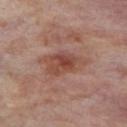{
  "biopsy_status": "not biopsied; imaged during a skin examination",
  "lesion_size": {
    "long_diameter_mm_approx": 4.5
  },
  "site": "leg",
  "lighting": "cross-polarized",
  "image": {
    "source": "total-body photography crop",
    "field_of_view_mm": 15
  },
  "patient": {
    "sex": "female",
    "age_approx": 55
  }
}